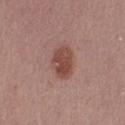Imaged during a routine full-body skin examination; the lesion was not biopsied and no histopathology is available.